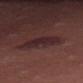Impression:
This lesion was catalogued during total-body skin photography and was not selected for biopsy.
Context:
Automated tile analysis of the lesion measured about 7 CIELAB-L* units darker than the surrounding skin and a normalized border contrast of about 8. The software also gave border irregularity of about 3 on a 0–10 scale, a within-lesion color-variation index near 3/10, and a peripheral color-asymmetry measure near 1. A 15 mm close-up tile from a total-body photography series done for melanoma screening. The subject is a female in their 30s. About 5 mm across. Imaged with white-light lighting. From the abdomen.